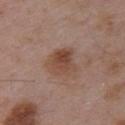{
  "biopsy_status": "not biopsied; imaged during a skin examination",
  "automated_metrics": {
    "area_mm2_approx": 10.0,
    "eccentricity": 0.4,
    "shape_asymmetry": 0.25,
    "border_irregularity_0_10": 3.0,
    "color_variation_0_10": 6.0,
    "peripheral_color_asymmetry": 2.5,
    "nevus_likeness_0_100": 10,
    "lesion_detection_confidence_0_100": 100
  },
  "lighting": "white-light",
  "patient": {
    "sex": "male",
    "age_approx": 55
  },
  "site": "right upper arm",
  "image": {
    "source": "total-body photography crop",
    "field_of_view_mm": 15
  }
}